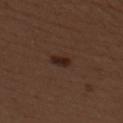Imaged during a routine full-body skin examination; the lesion was not biopsied and no histopathology is available.
Measured at roughly 2.5 mm in maximum diameter.
A lesion tile, about 15 mm wide, cut from a 3D total-body photograph.
The patient is a female approximately 50 years of age.
Imaged with white-light lighting.
The lesion is on the upper back.
The lesion-visualizer software estimated a footprint of about 3.5 mm² and a symmetry-axis asymmetry near 0.3. The analysis additionally found about 8 CIELAB-L* units darker than the surrounding skin and a lesion-to-skin contrast of about 9 (normalized; higher = more distinct). It also reported an automated nevus-likeness rating near 90 out of 100.